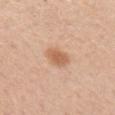From the arm.
Imaged with white-light lighting.
Cropped from a whole-body photographic skin survey; the tile spans about 15 mm.
A female subject about 45 years old.
An algorithmic analysis of the crop reported a lesion area of about 6 mm², an eccentricity of roughly 0.35, and a symmetry-axis asymmetry near 0.2. The analysis additionally found a lesion color around L≈62 a*≈21 b*≈34 in CIELAB, about 10 CIELAB-L* units darker than the surrounding skin, and a lesion-to-skin contrast of about 7 (normalized; higher = more distinct).